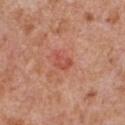Assessment:
No biopsy was performed on this lesion — it was imaged during a full skin examination and was not determined to be concerning.
Acquisition and patient details:
The patient is a male in their mid-60s. An algorithmic analysis of the crop reported a lesion area of about 4 mm², a shape eccentricity near 0.7, and a shape-asymmetry score of about 0.35 (0 = symmetric). And it measured a lesion color around L≈52 a*≈31 b*≈31 in CIELAB, a lesion–skin lightness drop of about 8, and a normalized border contrast of about 5.5. The analysis additionally found an automated nevus-likeness rating near 5 out of 100 and a detector confidence of about 100 out of 100 that the crop contains a lesion. The lesion is on the chest. The tile uses white-light illumination. A lesion tile, about 15 mm wide, cut from a 3D total-body photograph. About 2.5 mm across.